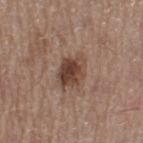A female patient, about 50 years old. Measured at roughly 4.5 mm in maximum diameter. A lesion tile, about 15 mm wide, cut from a 3D total-body photograph. Automated image analysis of the tile measured a footprint of about 9.5 mm², an eccentricity of roughly 0.7, and two-axis asymmetry of about 0.2. The analysis additionally found a mean CIELAB color near L≈43 a*≈18 b*≈25, about 12 CIELAB-L* units darker than the surrounding skin, and a lesion-to-skin contrast of about 9.5 (normalized; higher = more distinct). It also reported a peripheral color-asymmetry measure near 2.5. On the left thigh. Captured under white-light illumination.